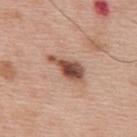This lesion was catalogued during total-body skin photography and was not selected for biopsy.
This is a white-light tile.
Located on the upper back.
A male patient about 60 years old.
Cropped from a whole-body photographic skin survey; the tile spans about 15 mm.
The lesion's longest dimension is about 4.5 mm.